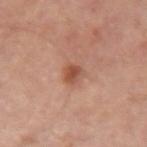Captured during whole-body skin photography for melanoma surveillance; the lesion was not biopsied. Automated image analysis of the tile measured an area of roughly 4 mm² and a shape eccentricity near 0.65. It also reported a lesion color around L≈50 a*≈24 b*≈31 in CIELAB and a lesion–skin lightness drop of about 10. And it measured an automated nevus-likeness rating near 85 out of 100 and a detector confidence of about 100 out of 100 that the crop contains a lesion. The lesion's longest dimension is about 2.5 mm. The lesion is located on the left upper arm. Cropped from a whole-body photographic skin survey; the tile spans about 15 mm. Captured under cross-polarized illumination. The patient is aged 53–57.